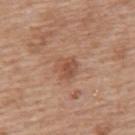Findings:
- notes: total-body-photography surveillance lesion; no biopsy